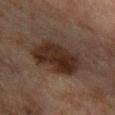Assessment: Part of a total-body skin-imaging series; this lesion was reviewed on a skin check and was not flagged for biopsy. Background: Cropped from a whole-body photographic skin survey; the tile spans about 15 mm. Located on the right forearm. A male subject approximately 45 years of age.